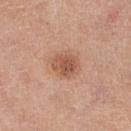Q: What is the lesion's diameter?
A: about 3.5 mm
Q: What is the imaging modality?
A: ~15 mm tile from a whole-body skin photo
Q: What are the patient's age and sex?
A: female, aged 63 to 67
Q: What lighting was used for the tile?
A: white-light
Q: What is the anatomic site?
A: the left leg